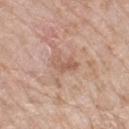follow-up: catalogued during a skin exam; not biopsied | TBP lesion metrics: an area of roughly 4.5 mm² and an outline eccentricity of about 0.8 (0 = round, 1 = elongated); a border-irregularity rating of about 4.5/10, a color-variation rating of about 3.5/10, and peripheral color asymmetry of about 1.5; a classifier nevus-likeness of about 0/100 and lesion-presence confidence of about 100/100 | lighting: white-light illumination | acquisition: ~15 mm crop, total-body skin-cancer survey | body site: the left lower leg | size: ≈3 mm | patient: female, aged around 70.